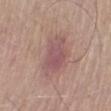Clinical impression:
Part of a total-body skin-imaging series; this lesion was reviewed on a skin check and was not flagged for biopsy.
Background:
From the right lower leg. A male patient, about 60 years old. Cropped from a total-body skin-imaging series; the visible field is about 15 mm.